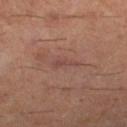Clinical impression: Captured during whole-body skin photography for melanoma surveillance; the lesion was not biopsied. Background: From the right thigh. Automated tile analysis of the lesion measured an area of roughly 3 mm², an outline eccentricity of about 0.95 (0 = round, 1 = elongated), and a symmetry-axis asymmetry near 0.45. A male patient, aged around 60. A 15 mm close-up extracted from a 3D total-body photography capture. This is a cross-polarized tile.